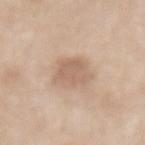Imaged during a routine full-body skin examination; the lesion was not biopsied and no histopathology is available. A female subject, in their 60s. Longest diameter approximately 3.5 mm. The total-body-photography lesion software estimated a lesion area of about 9 mm² and an eccentricity of roughly 0.55. The analysis additionally found a classifier nevus-likeness of about 15/100 and a detector confidence of about 100 out of 100 that the crop contains a lesion. On the mid back. A roughly 15 mm field-of-view crop from a total-body skin photograph.